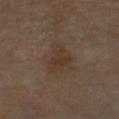| key | value |
|---|---|
| workup | total-body-photography surveillance lesion; no biopsy |
| acquisition | 15 mm crop, total-body photography |
| body site | the left upper arm |
| lighting | cross-polarized illumination |
| patient | male, about 70 years old |
| lesion size | ≈4 mm |